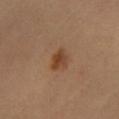biopsy status: no biopsy performed (imaged during a skin exam); site: the leg; tile lighting: cross-polarized illumination; diameter: ≈3 mm; subject: female, in their 60s; image source: ~15 mm crop, total-body skin-cancer survey.